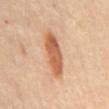Assessment: The lesion was photographed on a routine skin check and not biopsied; there is no pathology result. Background: This is a cross-polarized tile. The lesion is located on the mid back. Cropped from a total-body skin-imaging series; the visible field is about 15 mm. A female patient, aged around 60. The lesion-visualizer software estimated an average lesion color of about L≈60 a*≈25 b*≈37 (CIELAB), a lesion–skin lightness drop of about 13, and a lesion-to-skin contrast of about 9 (normalized; higher = more distinct). The lesion's longest dimension is about 6 mm.